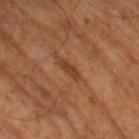Part of a total-body skin-imaging series; this lesion was reviewed on a skin check and was not flagged for biopsy.
Measured at roughly 3 mm in maximum diameter.
The total-body-photography lesion software estimated a border-irregularity rating of about 4/10, a within-lesion color-variation index near 0/10, and peripheral color asymmetry of about 0.
This is a cross-polarized tile.
The patient is a male aged 63 to 67.
Cropped from a whole-body photographic skin survey; the tile spans about 15 mm.